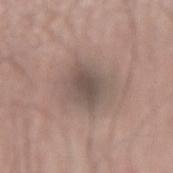  biopsy_status: not biopsied; imaged during a skin examination
  site: right forearm
  patient:
    sex: male
    age_approx: 65
  image:
    source: total-body photography crop
    field_of_view_mm: 15